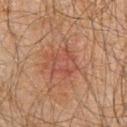Recorded during total-body skin imaging; not selected for excision or biopsy.
About 3.5 mm across.
On the chest.
A male patient approximately 60 years of age.
A region of skin cropped from a whole-body photographic capture, roughly 15 mm wide.